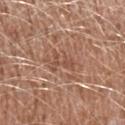– follow-up · no biopsy performed (imaged during a skin exam)
– subject · male, in their mid- to late 40s
– illumination · white-light
– acquisition · ~15 mm crop, total-body skin-cancer survey
– location · the left upper arm